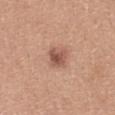Imaged during a routine full-body skin examination; the lesion was not biopsied and no histopathology is available. The lesion-visualizer software estimated a footprint of about 5.5 mm², a shape eccentricity near 0.5, and two-axis asymmetry of about 0.25. The software also gave a border-irregularity rating of about 2/10 and a color-variation rating of about 4.5/10. The software also gave a classifier nevus-likeness of about 85/100 and a lesion-detection confidence of about 100/100. The lesion is located on the back. Approximately 2.5 mm at its widest. A female patient, aged 28 to 32. Cropped from a whole-body photographic skin survey; the tile spans about 15 mm. The tile uses white-light illumination.